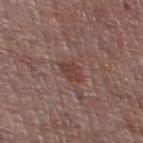Clinical impression:
The lesion was tiled from a total-body skin photograph and was not biopsied.